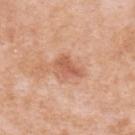Part of a total-body skin-imaging series; this lesion was reviewed on a skin check and was not flagged for biopsy. Measured at roughly 3 mm in maximum diameter. The patient is a female aged approximately 40. A region of skin cropped from a whole-body photographic capture, roughly 15 mm wide. The lesion is located on the upper back.